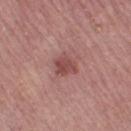The lesion was photographed on a routine skin check and not biopsied; there is no pathology result.
A lesion tile, about 15 mm wide, cut from a 3D total-body photograph.
Located on the right thigh.
The total-body-photography lesion software estimated an area of roughly 5.5 mm², a shape eccentricity near 0.6, and a shape-asymmetry score of about 0.25 (0 = symmetric). The software also gave a lesion color around L≈48 a*≈26 b*≈23 in CIELAB and a normalized border contrast of about 7.5. And it measured a classifier nevus-likeness of about 15/100.
The tile uses white-light illumination.
Approximately 3 mm at its widest.
A female patient approximately 55 years of age.